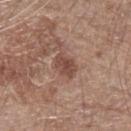This lesion was catalogued during total-body skin photography and was not selected for biopsy. Cropped from a whole-body photographic skin survey; the tile spans about 15 mm. The tile uses white-light illumination. Automated tile analysis of the lesion measured a lesion color around L≈47 a*≈21 b*≈25 in CIELAB, about 10 CIELAB-L* units darker than the surrounding skin, and a normalized border contrast of about 7.5. The analysis additionally found border irregularity of about 2.5 on a 0–10 scale, a color-variation rating of about 2.5/10, and peripheral color asymmetry of about 0.5. The analysis additionally found a nevus-likeness score of about 15/100 and lesion-presence confidence of about 100/100. About 3 mm across. On the left forearm. A male subject, roughly 75 years of age.